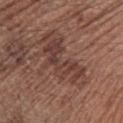| feature | finding |
|---|---|
| notes | total-body-photography surveillance lesion; no biopsy |
| anatomic site | the arm |
| subject | male, aged 68 to 72 |
| acquisition | 15 mm crop, total-body photography |